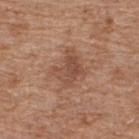{
  "biopsy_status": "not biopsied; imaged during a skin examination",
  "automated_metrics": {
    "area_mm2_approx": 10.0,
    "eccentricity": 0.55,
    "shape_asymmetry": 0.35,
    "border_irregularity_0_10": 5.0,
    "color_variation_0_10": 3.5,
    "peripheral_color_asymmetry": 1.5
  },
  "image": {
    "source": "total-body photography crop",
    "field_of_view_mm": 15
  },
  "patient": {
    "sex": "male",
    "age_approx": 65
  },
  "site": "upper back",
  "lesion_size": {
    "long_diameter_mm_approx": 4.0
  }
}